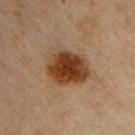No biopsy was performed on this lesion — it was imaged during a full skin examination and was not determined to be concerning. A roughly 15 mm field-of-view crop from a total-body skin photograph. Located on the chest. The subject is a male in their mid-40s. The tile uses cross-polarized illumination.